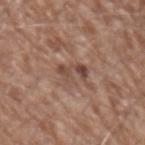| feature | finding |
|---|---|
| biopsy status | imaged on a skin check; not biopsied |
| patient | male, about 70 years old |
| illumination | white-light |
| size | ≈3 mm |
| image source | ~15 mm tile from a whole-body skin photo |
| anatomic site | the left forearm |
| image-analysis metrics | an automated nevus-likeness rating near 0 out of 100 |